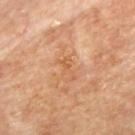| feature | finding |
|---|---|
| workup | total-body-photography surveillance lesion; no biopsy |
| site | the upper back |
| size | ≈3 mm |
| subject | male, in their mid-80s or older |
| image source | ~15 mm tile from a whole-body skin photo |
| tile lighting | cross-polarized |